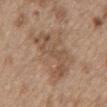notes — catalogued during a skin exam; not biopsied | location — the mid back | subject — male, in their 70s | image-analysis metrics — a shape-asymmetry score of about 0.55 (0 = symmetric); border irregularity of about 8.5 on a 0–10 scale and internal color variation of about 3 on a 0–10 scale; a nevus-likeness score of about 0/100 and lesion-presence confidence of about 100/100 | image source — ~15 mm crop, total-body skin-cancer survey | lesion diameter — ~6.5 mm (longest diameter).